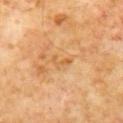The lesion was tiled from a total-body skin photograph and was not biopsied.
A 15 mm close-up tile from a total-body photography series done for melanoma screening.
This is a cross-polarized tile.
The recorded lesion diameter is about 2.5 mm.
The lesion is on the chest.
A male patient aged around 60.